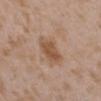Clinical impression:
The lesion was tiled from a total-body skin photograph and was not biopsied.
Image and clinical context:
A female patient, in their mid- to late 30s. The lesion is located on the arm. The tile uses white-light illumination. Automated tile analysis of the lesion measured a footprint of about 9 mm², an outline eccentricity of about 0.8 (0 = round, 1 = elongated), and a symmetry-axis asymmetry near 0.2. It also reported a mean CIELAB color near L≈53 a*≈18 b*≈31, roughly 9 lightness units darker than nearby skin, and a normalized lesion–skin contrast near 7.5. And it measured a border-irregularity rating of about 2.5/10, internal color variation of about 2.5 on a 0–10 scale, and a peripheral color-asymmetry measure near 1. And it measured an automated nevus-likeness rating near 5 out of 100 and lesion-presence confidence of about 100/100. The recorded lesion diameter is about 4 mm. A 15 mm close-up tile from a total-body photography series done for melanoma screening.